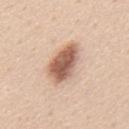Q: Was this lesion biopsied?
A: total-body-photography surveillance lesion; no biopsy
Q: How was this image acquired?
A: total-body-photography crop, ~15 mm field of view
Q: What did automated image analysis measure?
A: an area of roughly 12 mm² and two-axis asymmetry of about 0.2; a border-irregularity index near 2.5/10, a color-variation rating of about 5/10, and radial color variation of about 1.5; a nevus-likeness score of about 95/100
Q: What is the anatomic site?
A: the mid back
Q: What are the patient's age and sex?
A: male, about 45 years old